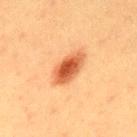* workup · catalogued during a skin exam; not biopsied
* imaging modality · total-body-photography crop, ~15 mm field of view
* lesion diameter · ~5 mm (longest diameter)
* subject · male, about 40 years old
* illumination · cross-polarized illumination
* location · the back